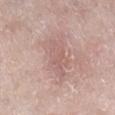workup: total-body-photography surveillance lesion; no biopsy | lesion size: ~5 mm (longest diameter) | site: the leg | tile lighting: white-light | subject: male, aged approximately 60 | image: ~15 mm tile from a whole-body skin photo | automated lesion analysis: a lesion area of about 12 mm², a shape eccentricity near 0.8, and a shape-asymmetry score of about 0.35 (0 = symmetric); a nevus-likeness score of about 0/100.